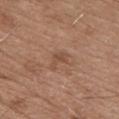Impression:
This lesion was catalogued during total-body skin photography and was not selected for biopsy.
Image and clinical context:
From the chest. The patient is a male aged around 65. Longest diameter approximately 3 mm. A lesion tile, about 15 mm wide, cut from a 3D total-body photograph.